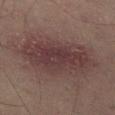follow-up: total-body-photography surveillance lesion; no biopsy
TBP lesion metrics: a lesion–skin lightness drop of about 8 and a lesion-to-skin contrast of about 8 (normalized; higher = more distinct); a lesion-detection confidence of about 100/100
size: ~9.5 mm (longest diameter)
patient: male, about 50 years old
illumination: cross-polarized illumination
location: the left thigh
imaging modality: ~15 mm tile from a whole-body skin photo